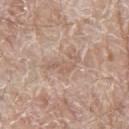This lesion was catalogued during total-body skin photography and was not selected for biopsy. A 15 mm crop from a total-body photograph taken for skin-cancer surveillance. A male subject, roughly 80 years of age. The lesion is located on the leg.